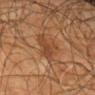This lesion was catalogued during total-body skin photography and was not selected for biopsy. A lesion tile, about 15 mm wide, cut from a 3D total-body photograph. Imaged with cross-polarized lighting. The patient is a male aged around 65. From the left upper arm.